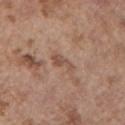Captured during whole-body skin photography for melanoma surveillance; the lesion was not biopsied. A female subject approximately 65 years of age. The lesion-visualizer software estimated a nevus-likeness score of about 0/100 and a lesion-detection confidence of about 90/100. A close-up tile cropped from a whole-body skin photograph, about 15 mm across. This is a white-light tile. The lesion is located on the left upper arm.